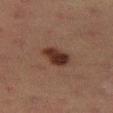This lesion was catalogued during total-body skin photography and was not selected for biopsy. A female patient, aged around 55. On the left thigh. The recorded lesion diameter is about 3.5 mm. Cropped from a total-body skin-imaging series; the visible field is about 15 mm.